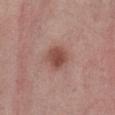{"lighting": "white-light", "automated_metrics": {"nevus_likeness_0_100": 95, "lesion_detection_confidence_0_100": 100}, "image": {"source": "total-body photography crop", "field_of_view_mm": 15}, "site": "abdomen", "patient": {"sex": "female", "age_approx": 55}}